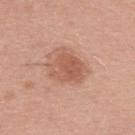<tbp_lesion>
  <biopsy_status>not biopsied; imaged during a skin examination</biopsy_status>
  <lesion_size>
    <long_diameter_mm_approx>4.5</long_diameter_mm_approx>
  </lesion_size>
  <automated_metrics>
    <nevus_likeness_0_100>70</nevus_likeness_0_100>
    <lesion_detection_confidence_0_100>100</lesion_detection_confidence_0_100>
  </automated_metrics>
  <image>
    <source>total-body photography crop</source>
    <field_of_view_mm>15</field_of_view_mm>
  </image>
  <patient>
    <sex>male</sex>
    <age_approx>45</age_approx>
  </patient>
  <site>upper back</site>
</tbp_lesion>